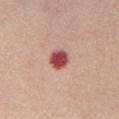The lesion was photographed on a routine skin check and not biopsied; there is no pathology result.
This is a white-light tile.
The lesion is on the chest.
The lesion's longest dimension is about 3 mm.
The subject is a female about 55 years old.
A lesion tile, about 15 mm wide, cut from a 3D total-body photograph.